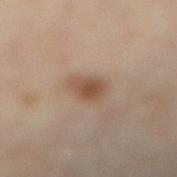Notes:
- tile lighting — cross-polarized
- location — the right lower leg
- patient — male, aged approximately 55
- imaging modality — ~15 mm tile from a whole-body skin photo